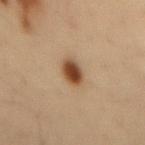Imaged during a routine full-body skin examination; the lesion was not biopsied and no histopathology is available.
The subject is a male in their mid- to late 30s.
Captured under cross-polarized illumination.
On the mid back.
A close-up tile cropped from a whole-body skin photograph, about 15 mm across.